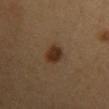From the front of the torso.
A close-up tile cropped from a whole-body skin photograph, about 15 mm across.
About 3 mm across.
The tile uses cross-polarized illumination.
A female subject, aged 28 to 32.